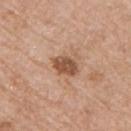Q: How large is the lesion?
A: ≈3.5 mm
Q: Where on the body is the lesion?
A: the chest
Q: Who is the patient?
A: male, aged 68 to 72
Q: What kind of image is this?
A: total-body-photography crop, ~15 mm field of view